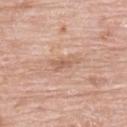Recorded during total-body skin imaging; not selected for excision or biopsy.
An algorithmic analysis of the crop reported an area of roughly 3.5 mm² and a symmetry-axis asymmetry near 0.45. The analysis additionally found a classifier nevus-likeness of about 0/100.
This image is a 15 mm lesion crop taken from a total-body photograph.
Longest diameter approximately 3.5 mm.
The lesion is on the upper back.
A female subject aged 68–72.